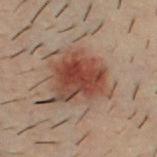Imaged during a routine full-body skin examination; the lesion was not biopsied and no histopathology is available.